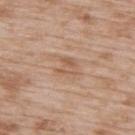Findings:
* workup · catalogued during a skin exam; not biopsied
* imaging modality · 15 mm crop, total-body photography
* automated lesion analysis · a lesion area of about 4.5 mm² and a shape-asymmetry score of about 0.45 (0 = symmetric); an average lesion color of about L≈57 a*≈19 b*≈31 (CIELAB) and a lesion-to-skin contrast of about 5.5 (normalized; higher = more distinct); border irregularity of about 4.5 on a 0–10 scale, a within-lesion color-variation index near 4/10, and peripheral color asymmetry of about 1.5; a nevus-likeness score of about 10/100 and a lesion-detection confidence of about 100/100
* patient · male, aged approximately 50
* diameter · ≈2.5 mm
* site · the upper back
* illumination · white-light illumination